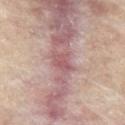Impression: Part of a total-body skin-imaging series; this lesion was reviewed on a skin check and was not flagged for biopsy. Image and clinical context: A male patient in their mid-80s or older. The recorded lesion diameter is about 3 mm. This is a cross-polarized tile. Cropped from a total-body skin-imaging series; the visible field is about 15 mm. Located on the abdomen. Automated image analysis of the tile measured a lesion color around L≈53 a*≈23 b*≈18 in CIELAB, about 9 CIELAB-L* units darker than the surrounding skin, and a lesion-to-skin contrast of about 6.5 (normalized; higher = more distinct). The software also gave border irregularity of about 3 on a 0–10 scale, internal color variation of about 2 on a 0–10 scale, and radial color variation of about 0.5.